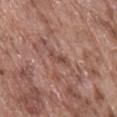Acquisition and patient details: The lesion is on the abdomen. The patient is a male in their mid- to late 70s. Measured at roughly 3 mm in maximum diameter. Captured under white-light illumination. A roughly 15 mm field-of-view crop from a total-body skin photograph.